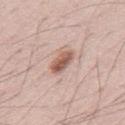Impression:
No biopsy was performed on this lesion — it was imaged during a full skin examination and was not determined to be concerning.
Clinical summary:
An algorithmic analysis of the crop reported a lesion area of about 6 mm², an eccentricity of roughly 0.8, and a symmetry-axis asymmetry near 0.25. The software also gave an automated nevus-likeness rating near 85 out of 100 and a detector confidence of about 100 out of 100 that the crop contains a lesion. A male subject aged around 70. Cropped from a total-body skin-imaging series; the visible field is about 15 mm. From the mid back. Captured under white-light illumination. About 3.5 mm across.